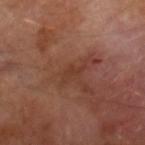Captured under cross-polarized illumination. The recorded lesion diameter is about 2.5 mm. From the right lower leg. A 15 mm crop from a total-body photograph taken for skin-cancer surveillance. The lesion-visualizer software estimated a lesion area of about 2.5 mm², a shape eccentricity near 0.85, and two-axis asymmetry of about 0.6. The software also gave a lesion color around L≈36 a*≈22 b*≈28 in CIELAB and a lesion–skin lightness drop of about 5. The software also gave border irregularity of about 6 on a 0–10 scale, a within-lesion color-variation index near 0/10, and a peripheral color-asymmetry measure near 0. It also reported a detector confidence of about 90 out of 100 that the crop contains a lesion. A male subject about 70 years old.